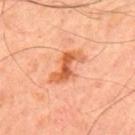The lesion was tiled from a total-body skin photograph and was not biopsied.
This is a cross-polarized tile.
On the upper back.
A 15 mm crop from a total-body photograph taken for skin-cancer surveillance.
A male patient, approximately 50 years of age.